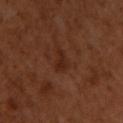workup: imaged on a skin check; not biopsied
image source: 15 mm crop, total-body photography
patient: male, approximately 50 years of age
size: about 2.5 mm
image-analysis metrics: an average lesion color of about L≈25 a*≈21 b*≈27 (CIELAB) and a normalized lesion–skin contrast near 6; a border-irregularity rating of about 3.5/10, a color-variation rating of about 1.5/10, and radial color variation of about 0.5
site: the back